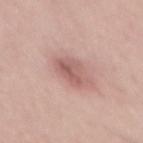<case>
  <biopsy_status>not biopsied; imaged during a skin examination</biopsy_status>
  <site>mid back</site>
  <patient>
    <sex>male</sex>
    <age_approx>25</age_approx>
  </patient>
  <lighting>white-light</lighting>
  <image>
    <source>total-body photography crop</source>
    <field_of_view_mm>15</field_of_view_mm>
  </image>
  <lesion_size>
    <long_diameter_mm_approx>4.0</long_diameter_mm_approx>
  </lesion_size>
</case>